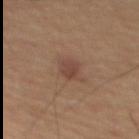{
  "biopsy_status": "not biopsied; imaged during a skin examination",
  "patient": {
    "sex": "male",
    "age_approx": 60
  },
  "site": "upper back",
  "lesion_size": {
    "long_diameter_mm_approx": 2.5
  },
  "image": {
    "source": "total-body photography crop",
    "field_of_view_mm": 15
  },
  "lighting": "cross-polarized"
}